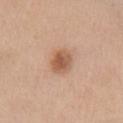Part of a total-body skin-imaging series; this lesion was reviewed on a skin check and was not flagged for biopsy.
From the chest.
A female subject roughly 55 years of age.
A 15 mm close-up tile from a total-body photography series done for melanoma screening.